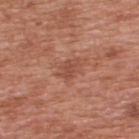size — about 2.5 mm
acquisition — total-body-photography crop, ~15 mm field of view
automated lesion analysis — an area of roughly 3 mm², a shape eccentricity near 0.8, and a symmetry-axis asymmetry near 0.35; a border-irregularity index near 3.5/10, a within-lesion color-variation index near 0.5/10, and peripheral color asymmetry of about 0.5
anatomic site — the upper back
tile lighting — white-light
patient — male, aged approximately 70